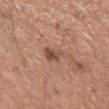Clinical impression: Imaged during a routine full-body skin examination; the lesion was not biopsied and no histopathology is available. Context: A male patient aged approximately 55. On the arm. Cropped from a total-body skin-imaging series; the visible field is about 15 mm.